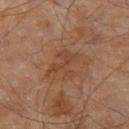Q: Was a biopsy performed?
A: total-body-photography surveillance lesion; no biopsy
Q: Illumination type?
A: cross-polarized illumination
Q: Who is the patient?
A: male, in their 60s
Q: What is the anatomic site?
A: the right leg
Q: How large is the lesion?
A: about 4.5 mm
Q: What is the imaging modality?
A: ~15 mm tile from a whole-body skin photo